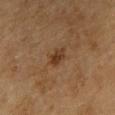This is a cross-polarized tile. A close-up tile cropped from a whole-body skin photograph, about 15 mm across. Approximately 2.5 mm at its widest. Automated image analysis of the tile measured a normalized border contrast of about 8. It also reported a border-irregularity rating of about 2.5/10 and peripheral color asymmetry of about 1. The analysis additionally found a classifier nevus-likeness of about 85/100 and lesion-presence confidence of about 100/100. The patient is a male aged approximately 60.